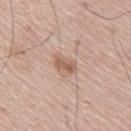<tbp_lesion>
  <biopsy_status>not biopsied; imaged during a skin examination</biopsy_status>
  <automated_metrics>
    <nevus_likeness_0_100>60</nevus_likeness_0_100>
  </automated_metrics>
  <patient>
    <sex>male</sex>
    <age_approx>75</age_approx>
  </patient>
  <image>
    <source>total-body photography crop</source>
    <field_of_view_mm>15</field_of_view_mm>
  </image>
  <lighting>white-light</lighting>
  <lesion_size>
    <long_diameter_mm_approx>2.5</long_diameter_mm_approx>
  </lesion_size>
  <site>upper back</site>
</tbp_lesion>